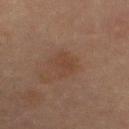This lesion was catalogued during total-body skin photography and was not selected for biopsy. Cropped from a whole-body photographic skin survey; the tile spans about 15 mm. The tile uses cross-polarized illumination. The lesion is on the left thigh. A female subject, in their 70s.